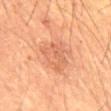This lesion was catalogued during total-body skin photography and was not selected for biopsy.